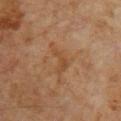  biopsy_status: not biopsied; imaged during a skin examination
  lighting: cross-polarized
  patient:
    sex: male
    age_approx: 60
  image:
    source: total-body photography crop
    field_of_view_mm: 15
  site: upper back